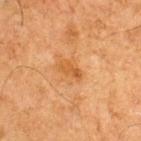Part of a total-body skin-imaging series; this lesion was reviewed on a skin check and was not flagged for biopsy. The lesion is on the upper back. A male patient approximately 65 years of age. A 15 mm close-up extracted from a 3D total-body photography capture.